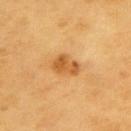Captured during whole-body skin photography for melanoma surveillance; the lesion was not biopsied. An algorithmic analysis of the crop reported an average lesion color of about L≈58 a*≈24 b*≈47 (CIELAB), roughly 11 lightness units darker than nearby skin, and a lesion-to-skin contrast of about 8 (normalized; higher = more distinct). It also reported a lesion-detection confidence of about 100/100. A close-up tile cropped from a whole-body skin photograph, about 15 mm across. The subject is a male aged 58–62. Imaged with cross-polarized lighting. From the upper back.